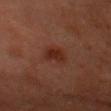Q: Was a biopsy performed?
A: no biopsy performed (imaged during a skin exam)
Q: How was the tile lit?
A: cross-polarized
Q: What is the imaging modality?
A: total-body-photography crop, ~15 mm field of view
Q: What are the patient's age and sex?
A: male, approximately 50 years of age
Q: Lesion size?
A: about 3 mm
Q: Lesion location?
A: the head or neck
Q: Automated lesion metrics?
A: a border-irregularity rating of about 3/10, a within-lesion color-variation index near 3/10, and peripheral color asymmetry of about 1; a nevus-likeness score of about 90/100 and lesion-presence confidence of about 100/100